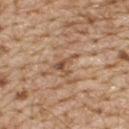{"biopsy_status": "not biopsied; imaged during a skin examination", "patient": {"sex": "male", "age_approx": 70}, "image": {"source": "total-body photography crop", "field_of_view_mm": 15}, "site": "upper back"}